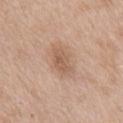No biopsy was performed on this lesion — it was imaged during a full skin examination and was not determined to be concerning.